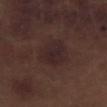<lesion>
  <biopsy_status>not biopsied; imaged during a skin examination</biopsy_status>
  <patient>
    <sex>male</sex>
    <age_approx>70</age_approx>
  </patient>
  <image>
    <source>total-body photography crop</source>
    <field_of_view_mm>15</field_of_view_mm>
  </image>
  <site>right lower leg</site>
</lesion>